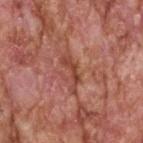| key | value |
|---|---|
| workup | imaged on a skin check; not biopsied |
| subject | male, approximately 60 years of age |
| site | the head or neck |
| TBP lesion metrics | a lesion area of about 4.5 mm², an eccentricity of roughly 0.95, and two-axis asymmetry of about 0.65; a border-irregularity rating of about 8/10 and radial color variation of about 0 |
| tile lighting | white-light illumination |
| image source | 15 mm crop, total-body photography |
| size | ~4.5 mm (longest diameter) |